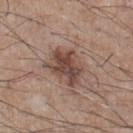Impression: The lesion was tiled from a total-body skin photograph and was not biopsied. Context: The lesion-visualizer software estimated a lesion–skin lightness drop of about 12 and a normalized border contrast of about 9. The analysis additionally found a color-variation rating of about 4.5/10. The software also gave a nevus-likeness score of about 50/100. A 15 mm crop from a total-body photograph taken for skin-cancer surveillance. Captured under white-light illumination. The lesion is located on the chest. A male subject aged 73 to 77.